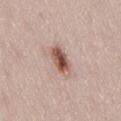{"automated_metrics": {"area_mm2_approx": 7.0, "eccentricity": 0.85, "shape_asymmetry": 0.2, "cielab_L": 54, "cielab_a": 20, "cielab_b": 26, "vs_skin_darker_L": 15.0, "vs_skin_contrast_norm": 10.0, "border_irregularity_0_10": 2.0, "color_variation_0_10": 7.5, "nevus_likeness_0_100": 95, "lesion_detection_confidence_0_100": 100}, "site": "back", "patient": {"sex": "female", "age_approx": 50}, "lesion_size": {"long_diameter_mm_approx": 4.0}, "image": {"source": "total-body photography crop", "field_of_view_mm": 15}, "lighting": "white-light"}